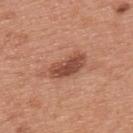Context:
A male patient in their mid- to late 50s. A region of skin cropped from a whole-body photographic capture, roughly 15 mm wide. On the upper back.